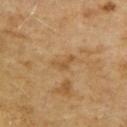Clinical impression: Imaged during a routine full-body skin examination; the lesion was not biopsied and no histopathology is available. Image and clinical context: On the right upper arm. A region of skin cropped from a whole-body photographic capture, roughly 15 mm wide. A female patient aged around 60.